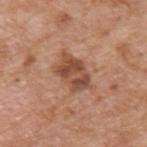Clinical impression:
Imaged during a routine full-body skin examination; the lesion was not biopsied and no histopathology is available.
Background:
A male subject, in their 60s. Automated tile analysis of the lesion measured a lesion area of about 11 mm², an eccentricity of roughly 0.75, and a shape-asymmetry score of about 0.2 (0 = symmetric). It also reported an average lesion color of about L≈49 a*≈23 b*≈31 (CIELAB), roughly 11 lightness units darker than nearby skin, and a lesion-to-skin contrast of about 8 (normalized; higher = more distinct). The software also gave a classifier nevus-likeness of about 15/100 and a lesion-detection confidence of about 100/100. Cropped from a whole-body photographic skin survey; the tile spans about 15 mm. The lesion is located on the upper back. Captured under white-light illumination.